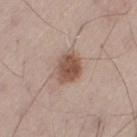follow-up: imaged on a skin check; not biopsied | anatomic site: the left thigh | size: ~4 mm (longest diameter) | image: ~15 mm tile from a whole-body skin photo | subject: male, aged approximately 55 | automated lesion analysis: an outline eccentricity of about 0.55 (0 = round, 1 = elongated) and two-axis asymmetry of about 0.2; border irregularity of about 1.5 on a 0–10 scale and peripheral color asymmetry of about 1.5; a classifier nevus-likeness of about 95/100 and a detector confidence of about 100 out of 100 that the crop contains a lesion.